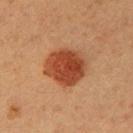Findings:
- biopsy status · catalogued during a skin exam; not biopsied
- body site · the right upper arm
- subject · male, in their 40s
- lesion size · ≈5 mm
- image source · ~15 mm crop, total-body skin-cancer survey
- lighting · cross-polarized illumination